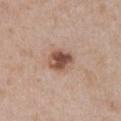  biopsy_status: not biopsied; imaged during a skin examination
  patient:
    sex: female
    age_approx: 30
  lesion_size:
    long_diameter_mm_approx: 3.0
  lighting: white-light
  automated_metrics:
    area_mm2_approx: 7.0
    eccentricity: 0.55
    shape_asymmetry: 0.15
    cielab_L: 51
    cielab_a: 20
    cielab_b: 27
    vs_skin_darker_L: 14.0
    vs_skin_contrast_norm: 10.0
    nevus_likeness_0_100: 90
    lesion_detection_confidence_0_100: 100
  site: chest
  image:
    source: total-body photography crop
    field_of_view_mm: 15